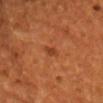The lesion was photographed on a routine skin check and not biopsied; there is no pathology result. A male subject aged 53–57. The recorded lesion diameter is about 2.5 mm. Captured under cross-polarized illumination. The lesion is located on the chest. A 15 mm close-up extracted from a 3D total-body photography capture.